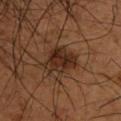notes: no biopsy performed (imaged during a skin exam); patient: male, aged around 50; image: total-body-photography crop, ~15 mm field of view; site: the back; lesion diameter: ~4 mm (longest diameter).